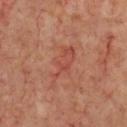Captured during whole-body skin photography for melanoma surveillance; the lesion was not biopsied.